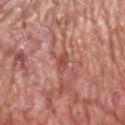Image and clinical context: Approximately 2.5 mm at its widest. Imaged with white-light lighting. A male patient aged approximately 60. On the head or neck. Cropped from a whole-body photographic skin survey; the tile spans about 15 mm.